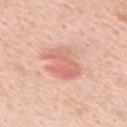image-analysis metrics = a footprint of about 12 mm² and a shape eccentricity near 0.7; an automated nevus-likeness rating near 40 out of 100 and a detector confidence of about 100 out of 100 that the crop contains a lesion | image = ~15 mm crop, total-body skin-cancer survey | location = the chest | patient = male, about 60 years old | lesion size = about 4.5 mm | tile lighting = white-light illumination.